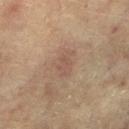Part of a total-body skin-imaging series; this lesion was reviewed on a skin check and was not flagged for biopsy. Longest diameter approximately 3 mm. This image is a 15 mm lesion crop taken from a total-body photograph. The total-body-photography lesion software estimated a lesion area of about 5 mm², an outline eccentricity of about 0.75 (0 = round, 1 = elongated), and two-axis asymmetry of about 0.25. The subject is a female roughly 80 years of age. From the left lower leg.